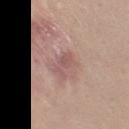{"biopsy_status": "not biopsied; imaged during a skin examination", "patient": {"sex": "female", "age_approx": 20}, "site": "left thigh", "lighting": "white-light", "lesion_size": {"long_diameter_mm_approx": 3.5}, "automated_metrics": {"area_mm2_approx": 5.0, "eccentricity": 0.85, "cielab_L": 55, "cielab_a": 21, "cielab_b": 22, "vs_skin_darker_L": 8.0, "vs_skin_contrast_norm": 6.0}, "image": {"source": "total-body photography crop", "field_of_view_mm": 15}}